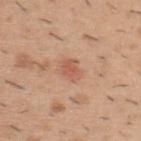Context: The lesion is on the upper back. Cropped from a total-body skin-imaging series; the visible field is about 15 mm. The subject is a male aged approximately 40. Approximately 3 mm at its widest. Captured under white-light illumination.